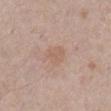This lesion was catalogued during total-body skin photography and was not selected for biopsy. Automated image analysis of the tile measured an area of roughly 5.5 mm² and a symmetry-axis asymmetry near 0.2. The analysis additionally found border irregularity of about 2 on a 0–10 scale, a color-variation rating of about 1.5/10, and a peripheral color-asymmetry measure near 0.5. Measured at roughly 3 mm in maximum diameter. The lesion is located on the front of the torso. Cropped from a whole-body photographic skin survey; the tile spans about 15 mm. The subject is a male aged 53 to 57. Captured under white-light illumination.